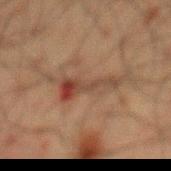<case>
  <biopsy_status>not biopsied; imaged during a skin examination</biopsy_status>
  <patient>
    <sex>male</sex>
    <age_approx>60</age_approx>
  </patient>
  <image>
    <source>total-body photography crop</source>
    <field_of_view_mm>15</field_of_view_mm>
  </image>
  <lesion_size>
    <long_diameter_mm_approx>7.5</long_diameter_mm_approx>
  </lesion_size>
  <automated_metrics>
    <area_mm2_approx>11.0</area_mm2_approx>
    <eccentricity>0.95</eccentricity>
    <border_irregularity_0_10>9.0</border_irregularity_0_10>
    <nevus_likeness_0_100>0</nevus_likeness_0_100>
    <lesion_detection_confidence_0_100>65</lesion_detection_confidence_0_100>
  </automated_metrics>
  <site>mid back</site>
</case>